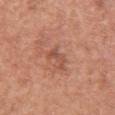Assessment:
Part of a total-body skin-imaging series; this lesion was reviewed on a skin check and was not flagged for biopsy.
Context:
A lesion tile, about 15 mm wide, cut from a 3D total-body photograph. About 3 mm across. The subject is a female aged 58 to 62. Captured under white-light illumination. Automated image analysis of the tile measured a lesion color around L≈52 a*≈25 b*≈30 in CIELAB, about 8 CIELAB-L* units darker than the surrounding skin, and a normalized border contrast of about 6. And it measured a within-lesion color-variation index near 0/10 and a peripheral color-asymmetry measure near 0. The lesion is located on the upper back.